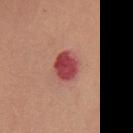| field | value |
|---|---|
| workup | no biopsy performed (imaged during a skin exam) |